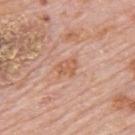Assessment:
No biopsy was performed on this lesion — it was imaged during a full skin examination and was not determined to be concerning.
Context:
Approximately 2.5 mm at its widest. Cropped from a total-body skin-imaging series; the visible field is about 15 mm. The subject is a male approximately 80 years of age. Located on the back.